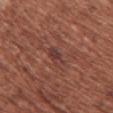Assessment:
Part of a total-body skin-imaging series; this lesion was reviewed on a skin check and was not flagged for biopsy.
Background:
Captured under white-light illumination. Automated image analysis of the tile measured an area of roughly 4 mm², an outline eccentricity of about 0.9 (0 = round, 1 = elongated), and a symmetry-axis asymmetry near 0.35. And it measured an average lesion color of about L≈38 a*≈23 b*≈23 (CIELAB) and a lesion-to-skin contrast of about 7 (normalized; higher = more distinct). The software also gave a classifier nevus-likeness of about 0/100 and lesion-presence confidence of about 90/100. A region of skin cropped from a whole-body photographic capture, roughly 15 mm wide. Approximately 3 mm at its widest. A female subject, aged 63–67. On the chest.